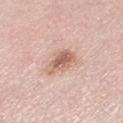{"biopsy_status": "not biopsied; imaged during a skin examination", "site": "right thigh", "image": {"source": "total-body photography crop", "field_of_view_mm": 15}, "patient": {"sex": "female", "age_approx": 65}}